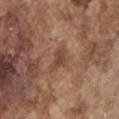The lesion was tiled from a total-body skin photograph and was not biopsied. Cropped from a whole-body photographic skin survey; the tile spans about 15 mm. The lesion-visualizer software estimated an average lesion color of about L≈45 a*≈20 b*≈29 (CIELAB), about 8 CIELAB-L* units darker than the surrounding skin, and a lesion-to-skin contrast of about 6.5 (normalized; higher = more distinct). And it measured border irregularity of about 3.5 on a 0–10 scale and internal color variation of about 2 on a 0–10 scale. The tile uses white-light illumination. A male patient, in their mid- to late 70s. Located on the chest. The recorded lesion diameter is about 3 mm.